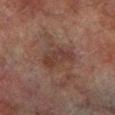follow-up — catalogued during a skin exam; not biopsied
lesion diameter — about 4.5 mm
image source — ~15 mm crop, total-body skin-cancer survey
lighting — cross-polarized
patient — male, aged 73 to 77
site — the left lower leg